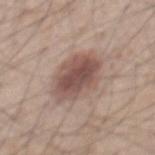Longest diameter approximately 6.5 mm. The lesion is located on the mid back. A male patient, aged 43 to 47. Cropped from a whole-body photographic skin survey; the tile spans about 15 mm. Imaged with white-light lighting.